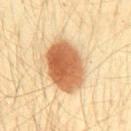notes = no biopsy performed (imaged during a skin exam) | automated metrics = a mean CIELAB color near L≈61 a*≈23 b*≈40 and a normalized border contrast of about 11; a within-lesion color-variation index near 5.5/10 and radial color variation of about 1.5 | location = the front of the torso | lesion size = ~6 mm (longest diameter) | subject = male, aged approximately 30 | image = 15 mm crop, total-body photography.